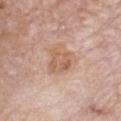The lesion was tiled from a total-body skin photograph and was not biopsied.
Located on the chest.
A male patient aged 83 or older.
A 15 mm close-up tile from a total-body photography series done for melanoma screening.